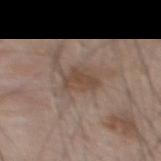Assessment: Part of a total-body skin-imaging series; this lesion was reviewed on a skin check and was not flagged for biopsy. Context: This is a white-light tile. The patient is a male aged 48 to 52. Automated image analysis of the tile measured a mean CIELAB color near L≈46 a*≈15 b*≈25, a lesion–skin lightness drop of about 8, and a normalized lesion–skin contrast near 6.5. It also reported a within-lesion color-variation index near 3/10 and a peripheral color-asymmetry measure near 1. From the mid back. The recorded lesion diameter is about 3.5 mm. A region of skin cropped from a whole-body photographic capture, roughly 15 mm wide.